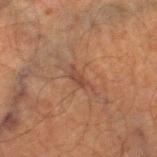Recorded during total-body skin imaging; not selected for excision or biopsy.